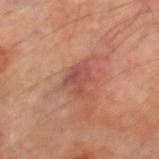biopsy_status: not biopsied; imaged during a skin examination
automated_metrics:
  cielab_L: 49
  cielab_a: 25
  cielab_b: 27
  vs_skin_darker_L: 8.0
  vs_skin_contrast_norm: 6.0
  border_irregularity_0_10: 5.0
  color_variation_0_10: 3.5
  peripheral_color_asymmetry: 1.0
lighting: cross-polarized
site: right thigh
lesion_size:
  long_diameter_mm_approx: 3.5
patient:
  sex: male
  age_approx: 70
image:
  source: total-body photography crop
  field_of_view_mm: 15